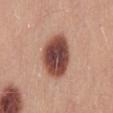Part of a total-body skin-imaging series; this lesion was reviewed on a skin check and was not flagged for biopsy.
The total-body-photography lesion software estimated a shape eccentricity near 0.7 and two-axis asymmetry of about 0.15. It also reported an average lesion color of about L≈46 a*≈24 b*≈25 (CIELAB), roughly 20 lightness units darker than nearby skin, and a lesion-to-skin contrast of about 13.5 (normalized; higher = more distinct). It also reported a border-irregularity index near 1/10, a color-variation rating of about 7/10, and a peripheral color-asymmetry measure near 1.5.
Captured under white-light illumination.
The recorded lesion diameter is about 5.5 mm.
A male subject, in their mid-20s.
A lesion tile, about 15 mm wide, cut from a 3D total-body photograph.
On the mid back.